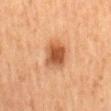Impression:
Imaged during a routine full-body skin examination; the lesion was not biopsied and no histopathology is available.
Image and clinical context:
An algorithmic analysis of the crop reported a footprint of about 9.5 mm², an eccentricity of roughly 0.55, and a symmetry-axis asymmetry near 0.15. The software also gave a mean CIELAB color near L≈44 a*≈22 b*≈32, a lesion–skin lightness drop of about 12, and a normalized lesion–skin contrast near 9. The software also gave a color-variation rating of about 5/10 and radial color variation of about 1.5. And it measured a lesion-detection confidence of about 100/100. Located on the mid back. A female subject, aged 58–62. A close-up tile cropped from a whole-body skin photograph, about 15 mm across. Measured at roughly 3.5 mm in maximum diameter.